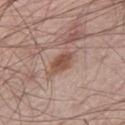No biopsy was performed on this lesion — it was imaged during a full skin examination and was not determined to be concerning.
A male patient, in their mid-60s.
A close-up tile cropped from a whole-body skin photograph, about 15 mm across.
The lesion is on the right thigh.
The total-body-photography lesion software estimated a lesion–skin lightness drop of about 11. The software also gave a lesion-detection confidence of about 100/100.
Longest diameter approximately 3.5 mm.
The tile uses white-light illumination.